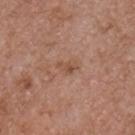Q: Is there a histopathology result?
A: total-body-photography surveillance lesion; no biopsy
Q: Automated lesion metrics?
A: a mean CIELAB color near L≈51 a*≈21 b*≈30, roughly 7 lightness units darker than nearby skin, and a normalized lesion–skin contrast near 5.5; border irregularity of about 3.5 on a 0–10 scale, internal color variation of about 3 on a 0–10 scale, and radial color variation of about 1.5
Q: Patient demographics?
A: male, aged 53 to 57
Q: How large is the lesion?
A: about 2.5 mm
Q: Where on the body is the lesion?
A: the chest
Q: What is the imaging modality?
A: ~15 mm tile from a whole-body skin photo
Q: Illumination type?
A: white-light